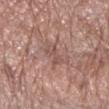| field | value |
|---|---|
| biopsy status | no biopsy performed (imaged during a skin exam) |
| patient | male, aged around 70 |
| lesion size | ≈3 mm |
| TBP lesion metrics | an area of roughly 4 mm², an outline eccentricity of about 0.85 (0 = round, 1 = elongated), and two-axis asymmetry of about 0.35; a mean CIELAB color near L≈52 a*≈20 b*≈24, a lesion–skin lightness drop of about 7, and a lesion-to-skin contrast of about 5 (normalized; higher = more distinct); a border-irregularity index near 4.5/10, a within-lesion color-variation index near 1/10, and a peripheral color-asymmetry measure near 0.5 |
| anatomic site | the right forearm |
| image | 15 mm crop, total-body photography |